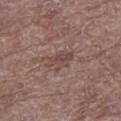notes = total-body-photography surveillance lesion; no biopsy | acquisition = ~15 mm crop, total-body skin-cancer survey | lesion diameter = ≈4 mm | patient = male, aged approximately 70 | tile lighting = white-light illumination | automated lesion analysis = a color-variation rating of about 2/10 and peripheral color asymmetry of about 0.5; a nevus-likeness score of about 0/100 | body site = the left lower leg.